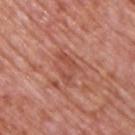{"site": "upper back", "image": {"source": "total-body photography crop", "field_of_view_mm": 15}, "lighting": "white-light", "patient": {"sex": "male", "age_approx": 75}, "automated_metrics": {"area_mm2_approx": 5.0, "eccentricity": 0.85, "shape_asymmetry": 0.55, "border_irregularity_0_10": 8.0, "color_variation_0_10": 0.0, "peripheral_color_asymmetry": 0.0, "nevus_likeness_0_100": 0, "lesion_detection_confidence_0_100": 100}, "lesion_size": {"long_diameter_mm_approx": 4.0}}